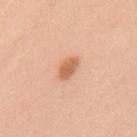notes: no biopsy performed (imaged during a skin exam); image: total-body-photography crop, ~15 mm field of view; patient: female, aged 38 to 42; illumination: white-light illumination; lesion size: ~3 mm (longest diameter); body site: the upper back.